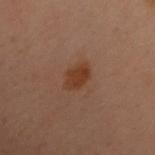  biopsy_status: not biopsied; imaged during a skin examination
  site: back
  patient:
    sex: female
    age_approx: 60
  lighting: cross-polarized
  image:
    source: total-body photography crop
    field_of_view_mm: 15
  lesion_size:
    long_diameter_mm_approx: 3.5
  automated_metrics:
    area_mm2_approx: 6.0
    eccentricity: 0.8
    shape_asymmetry: 0.2
    cielab_L: 32
    cielab_a: 19
    cielab_b: 27
    vs_skin_darker_L: 7.0
    vs_skin_contrast_norm: 8.5